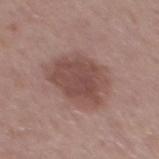  biopsy_status: not biopsied; imaged during a skin examination
  image:
    source: total-body photography crop
    field_of_view_mm: 15
  automated_metrics:
    border_irregularity_0_10: 3.0
    peripheral_color_asymmetry: 1.0
    lesion_detection_confidence_0_100: 100
  lighting: white-light
  patient:
    sex: male
    age_approx: 60
  site: mid back
  lesion_size:
    long_diameter_mm_approx: 6.0